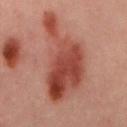The lesion was photographed on a routine skin check and not biopsied; there is no pathology result. The recorded lesion diameter is about 11 mm. A male patient aged 38–42. A close-up tile cropped from a whole-body skin photograph, about 15 mm across. On the mid back. Captured under cross-polarized illumination.